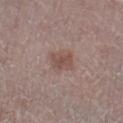The lesion was tiled from a total-body skin photograph and was not biopsied. A female subject aged approximately 65. About 3 mm across. The tile uses white-light illumination. The lesion-visualizer software estimated a mean CIELAB color near L≈49 a*≈18 b*≈22, roughly 8 lightness units darker than nearby skin, and a lesion-to-skin contrast of about 6.5 (normalized; higher = more distinct). The analysis additionally found a border-irregularity rating of about 2/10, internal color variation of about 2 on a 0–10 scale, and a peripheral color-asymmetry measure near 0.5. The software also gave a nevus-likeness score of about 40/100 and a detector confidence of about 100 out of 100 that the crop contains a lesion. This image is a 15 mm lesion crop taken from a total-body photograph. From the left leg.